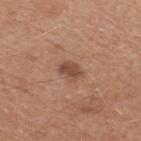Captured during whole-body skin photography for melanoma surveillance; the lesion was not biopsied. The lesion is on the upper back. A male patient, aged 63 to 67. A roughly 15 mm field-of-view crop from a total-body skin photograph.